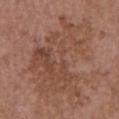follow-up = catalogued during a skin exam; not biopsied | image-analysis metrics = a border-irregularity index near 9/10, a color-variation rating of about 5/10, and peripheral color asymmetry of about 2; a nevus-likeness score of about 0/100 and lesion-presence confidence of about 100/100 | image = ~15 mm tile from a whole-body skin photo | diameter = ≈10.5 mm | site = the chest | patient = female, aged 63 to 67 | tile lighting = white-light illumination.